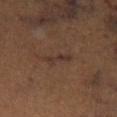Assessment:
Captured during whole-body skin photography for melanoma surveillance; the lesion was not biopsied.
Image and clinical context:
A 15 mm close-up extracted from a 3D total-body photography capture. The recorded lesion diameter is about 4 mm. On the left lower leg. A female subject in their mid- to late 60s. Automated image analysis of the tile measured an average lesion color of about L≈31 a*≈16 b*≈21 (CIELAB), roughly 5 lightness units darker than nearby skin, and a lesion-to-skin contrast of about 6 (normalized; higher = more distinct). This is a cross-polarized tile.